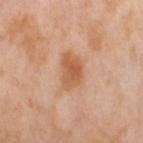Q: Was this lesion biopsied?
A: catalogued during a skin exam; not biopsied
Q: What is the anatomic site?
A: the leg
Q: How was the tile lit?
A: cross-polarized
Q: What did automated image analysis measure?
A: a border-irregularity index near 2.5/10 and a color-variation rating of about 3.5/10; a classifier nevus-likeness of about 20/100 and lesion-presence confidence of about 100/100
Q: What are the patient's age and sex?
A: female, aged around 55
Q: How large is the lesion?
A: ~4.5 mm (longest diameter)
Q: How was this image acquired?
A: ~15 mm tile from a whole-body skin photo